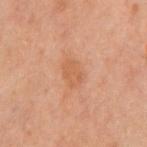Q: Is there a histopathology result?
A: catalogued during a skin exam; not biopsied
Q: What is the imaging modality?
A: ~15 mm tile from a whole-body skin photo
Q: Where on the body is the lesion?
A: the chest
Q: Lesion size?
A: ≈3 mm
Q: Patient demographics?
A: male, aged approximately 60